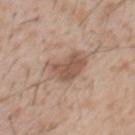Impression: The lesion was photographed on a routine skin check and not biopsied; there is no pathology result. Clinical summary: On the front of the torso. Cropped from a total-body skin-imaging series; the visible field is about 15 mm. The recorded lesion diameter is about 5.5 mm. The patient is a male aged around 45.